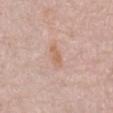Q: Is there a histopathology result?
A: no biopsy performed (imaged during a skin exam)
Q: What are the patient's age and sex?
A: male, roughly 80 years of age
Q: Where on the body is the lesion?
A: the chest
Q: Illumination type?
A: white-light
Q: Automated lesion metrics?
A: an area of roughly 4 mm², an outline eccentricity of about 0.9 (0 = round, 1 = elongated), and a symmetry-axis asymmetry near 0.3; an average lesion color of about L≈63 a*≈20 b*≈30 (CIELAB), a lesion–skin lightness drop of about 7, and a normalized lesion–skin contrast near 6; border irregularity of about 3.5 on a 0–10 scale, internal color variation of about 1 on a 0–10 scale, and radial color variation of about 0
Q: How was this image acquired?
A: ~15 mm tile from a whole-body skin photo